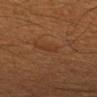This lesion was catalogued during total-body skin photography and was not selected for biopsy. The tile uses cross-polarized illumination. Automated image analysis of the tile measured an area of roughly 2.5 mm² and two-axis asymmetry of about 0.35. It also reported a mean CIELAB color near L≈32 a*≈20 b*≈29. The analysis additionally found a border-irregularity rating of about 3.5/10, internal color variation of about 0 on a 0–10 scale, and radial color variation of about 0. It also reported an automated nevus-likeness rating near 0 out of 100 and a detector confidence of about 100 out of 100 that the crop contains a lesion. The recorded lesion diameter is about 2.5 mm. A male subject about 60 years old. On the left forearm. A region of skin cropped from a whole-body photographic capture, roughly 15 mm wide.